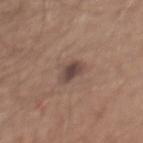The lesion was photographed on a routine skin check and not biopsied; there is no pathology result. On the mid back. A male subject aged 63 to 67. A lesion tile, about 15 mm wide, cut from a 3D total-body photograph.